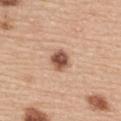  biopsy_status: not biopsied; imaged during a skin examination
  lesion_size:
    long_diameter_mm_approx: 2.5
  image:
    source: total-body photography crop
    field_of_view_mm: 15
  site: back
  lighting: white-light
  automated_metrics:
    cielab_L: 54
    cielab_a: 22
    cielab_b: 30
    vs_skin_darker_L: 16.0
    vs_skin_contrast_norm: 10.5
  patient:
    sex: female
    age_approx: 65